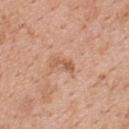Case summary:
* follow-up · no biopsy performed (imaged during a skin exam)
* subject · female, in their 50s
* TBP lesion metrics · a lesion area of about 2.5 mm², a shape eccentricity near 0.9, and two-axis asymmetry of about 0.5; a lesion color around L≈57 a*≈23 b*≈33 in CIELAB and about 10 CIELAB-L* units darker than the surrounding skin; a nevus-likeness score of about 0/100 and a lesion-detection confidence of about 100/100
* lighting · white-light
* acquisition · 15 mm crop, total-body photography
* size · about 2.5 mm
* location · the back